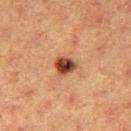Q: Was a biopsy performed?
A: imaged on a skin check; not biopsied
Q: What kind of image is this?
A: total-body-photography crop, ~15 mm field of view
Q: Lesion location?
A: the leg
Q: Who is the patient?
A: female, approximately 40 years of age
Q: Lesion size?
A: about 2.5 mm
Q: What lighting was used for the tile?
A: cross-polarized illumination